A roughly 15 mm field-of-view crop from a total-body skin photograph; the patient is a male about 55 years old; located on the chest — 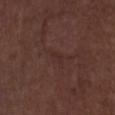Approximately 2 mm at its widest. Captured under white-light illumination. Histopathologically confirmed as a nodular basal cell carcinoma.